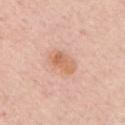Impression: Part of a total-body skin-imaging series; this lesion was reviewed on a skin check and was not flagged for biopsy. Background: Located on the chest. The lesion-visualizer software estimated a footprint of about 7.5 mm², an outline eccentricity of about 0.85 (0 = round, 1 = elongated), and two-axis asymmetry of about 0.2. The software also gave border irregularity of about 2 on a 0–10 scale, a within-lesion color-variation index near 4/10, and radial color variation of about 1.5. The analysis additionally found a classifier nevus-likeness of about 45/100 and a lesion-detection confidence of about 100/100. A male subject, aged approximately 65. The lesion's longest dimension is about 4 mm. A region of skin cropped from a whole-body photographic capture, roughly 15 mm wide.